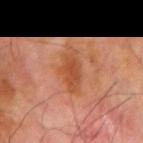Findings:
• biopsy status — total-body-photography surveillance lesion; no biopsy
• lighting — cross-polarized illumination
• acquisition — ~15 mm tile from a whole-body skin photo
• site — the left forearm
• image-analysis metrics — a lesion area of about 8.5 mm², an outline eccentricity of about 0.8 (0 = round, 1 = elongated), and two-axis asymmetry of about 0.35; about 9 CIELAB-L* units darker than the surrounding skin and a lesion-to-skin contrast of about 7 (normalized; higher = more distinct); an automated nevus-likeness rating near 60 out of 100
• subject — male, aged approximately 70
• size — ~4 mm (longest diameter)